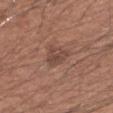Imaged during a routine full-body skin examination; the lesion was not biopsied and no histopathology is available.
Measured at roughly 3 mm in maximum diameter.
This is a white-light tile.
A male subject, aged 48–52.
A lesion tile, about 15 mm wide, cut from a 3D total-body photograph.
From the left forearm.